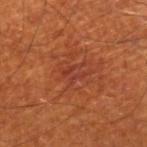– lesion diameter — about 3 mm
– TBP lesion metrics — a normalized lesion–skin contrast near 5.5; a border-irregularity rating of about 4.5/10, a within-lesion color-variation index near 2/10, and a peripheral color-asymmetry measure near 0.5
– patient — male, in their 70s
– acquisition — 15 mm crop, total-body photography
– illumination — cross-polarized
– body site — the leg